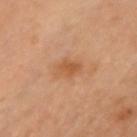{"biopsy_status": "not biopsied; imaged during a skin examination"}